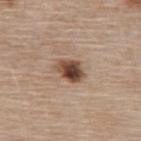This lesion was catalogued during total-body skin photography and was not selected for biopsy. On the upper back. Automated image analysis of the tile measured a symmetry-axis asymmetry near 0.15. The software also gave a border-irregularity rating of about 2/10, internal color variation of about 9.5 on a 0–10 scale, and peripheral color asymmetry of about 3. The patient is a female aged 63–67. Measured at roughly 3.5 mm in maximum diameter. A close-up tile cropped from a whole-body skin photograph, about 15 mm across.